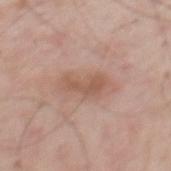Part of a total-body skin-imaging series; this lesion was reviewed on a skin check and was not flagged for biopsy. A 15 mm close-up extracted from a 3D total-body photography capture. A male patient aged 58–62. The lesion is on the mid back.